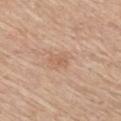Assessment:
Part of a total-body skin-imaging series; this lesion was reviewed on a skin check and was not flagged for biopsy.
Image and clinical context:
A 15 mm close-up tile from a total-body photography series done for melanoma screening. A female patient aged approximately 65. On the mid back. Captured under white-light illumination.